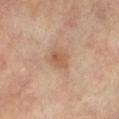Assessment:
This lesion was catalogued during total-body skin photography and was not selected for biopsy.
Background:
A female subject about 65 years old. Cropped from a total-body skin-imaging series; the visible field is about 15 mm. Measured at roughly 3 mm in maximum diameter. Located on the leg.